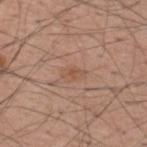Impression:
No biopsy was performed on this lesion — it was imaged during a full skin examination and was not determined to be concerning.
Background:
A close-up tile cropped from a whole-body skin photograph, about 15 mm across. The lesion is on the upper back. The lesion-visualizer software estimated an average lesion color of about L≈53 a*≈20 b*≈30 (CIELAB), a lesion–skin lightness drop of about 6, and a normalized lesion–skin contrast near 5.5. The software also gave a within-lesion color-variation index near 1/10 and a peripheral color-asymmetry measure near 0.5. It also reported a classifier nevus-likeness of about 0/100 and lesion-presence confidence of about 100/100. Imaged with white-light lighting. A male patient, approximately 60 years of age. Approximately 2.5 mm at its widest.